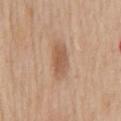lighting: white-light
site: mid back
image:
  source: total-body photography crop
  field_of_view_mm: 15
automated_metrics:
  area_mm2_approx: 7.5
  eccentricity: 0.85
  shape_asymmetry: 0.2
  cielab_L: 57
  cielab_a: 19
  cielab_b: 32
  vs_skin_darker_L: 10.0
  vs_skin_contrast_norm: 7.0
  border_irregularity_0_10: 2.5
  color_variation_0_10: 2.5
  peripheral_color_asymmetry: 1.0
  lesion_detection_confidence_0_100: 100
patient:
  sex: male
  age_approx: 60
lesion_size:
  long_diameter_mm_approx: 4.0